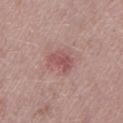Context: The lesion is located on the left lower leg. A male patient, aged 38 to 42. A close-up tile cropped from a whole-body skin photograph, about 15 mm across.